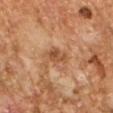Case summary:
• follow-up: no biopsy performed (imaged during a skin exam)
• imaging modality: 15 mm crop, total-body photography
• lighting: cross-polarized illumination
• subject: male, in their 60s
• anatomic site: the right forearm
• diameter: about 3 mm